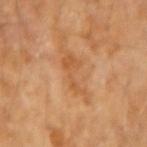The tile uses cross-polarized illumination.
A 15 mm close-up tile from a total-body photography series done for melanoma screening.
About 5.5 mm across.
The lesion is located on the left forearm.
An algorithmic analysis of the crop reported a mean CIELAB color near L≈54 a*≈23 b*≈39, about 7 CIELAB-L* units darker than the surrounding skin, and a normalized border contrast of about 5.5.
The subject is a male in their mid- to late 80s or older.